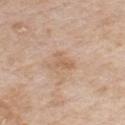{"biopsy_status": "not biopsied; imaged during a skin examination", "patient": {"sex": "male", "age_approx": 65}, "site": "front of the torso", "lighting": "white-light", "image": {"source": "total-body photography crop", "field_of_view_mm": 15}}